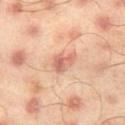<record>
<biopsy_status>not biopsied; imaged during a skin examination</biopsy_status>
<image>
  <source>total-body photography crop</source>
  <field_of_view_mm>15</field_of_view_mm>
</image>
<lesion_size>
  <long_diameter_mm_approx>2.5</long_diameter_mm_approx>
</lesion_size>
<patient>
  <sex>male</sex>
  <age_approx>45</age_approx>
</patient>
<lighting>cross-polarized</lighting>
<site>leg</site>
</record>